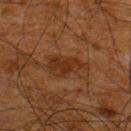{"biopsy_status": "not biopsied; imaged during a skin examination", "site": "back", "patient": {"sex": "male", "age_approx": 65}, "image": {"source": "total-body photography crop", "field_of_view_mm": 15}}